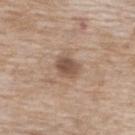Imaged during a routine full-body skin examination; the lesion was not biopsied and no histopathology is available. A roughly 15 mm field-of-view crop from a total-body skin photograph. Located on the upper back. The lesion-visualizer software estimated an average lesion color of about L≈51 a*≈17 b*≈27 (CIELAB), about 12 CIELAB-L* units darker than the surrounding skin, and a normalized border contrast of about 8.5. And it measured internal color variation of about 3.5 on a 0–10 scale. A female patient aged 73 to 77. The lesion's longest dimension is about 3 mm.